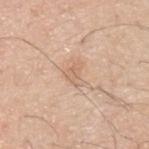The lesion was photographed on a routine skin check and not biopsied; there is no pathology result.
A male patient, approximately 30 years of age.
The tile uses white-light illumination.
Approximately 3 mm at its widest.
The total-body-photography lesion software estimated an area of roughly 4.5 mm², an eccentricity of roughly 0.65, and a symmetry-axis asymmetry near 0.5. It also reported a lesion color around L≈65 a*≈18 b*≈31 in CIELAB and a normalized border contrast of about 5. It also reported a border-irregularity rating of about 5/10, a color-variation rating of about 1.5/10, and peripheral color asymmetry of about 0.5. It also reported a lesion-detection confidence of about 100/100.
A roughly 15 mm field-of-view crop from a total-body skin photograph.
The lesion is located on the upper back.